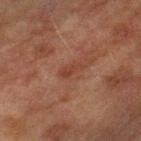Case summary:
• workup: imaged on a skin check; not biopsied
• subject: male, aged around 75
• site: the left forearm
• tile lighting: cross-polarized
• image: ~15 mm tile from a whole-body skin photo